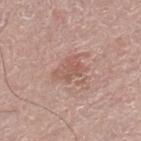biopsy status=no biopsy performed (imaged during a skin exam)
automated lesion analysis=an eccentricity of roughly 0.7; border irregularity of about 4.5 on a 0–10 scale and a peripheral color-asymmetry measure near 1; a classifier nevus-likeness of about 0/100 and lesion-presence confidence of about 100/100
location=the right lower leg
image source=~15 mm tile from a whole-body skin photo
subject=male, approximately 70 years of age
illumination=white-light illumination
diameter=≈3 mm